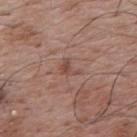follow-up — catalogued during a skin exam; not biopsied | site — the upper back | patient — male, about 70 years old | image source — ~15 mm crop, total-body skin-cancer survey.